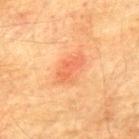The lesion was photographed on a routine skin check and not biopsied; there is no pathology result.
The tile uses cross-polarized illumination.
A region of skin cropped from a whole-body photographic capture, roughly 15 mm wide.
Longest diameter approximately 4 mm.
The lesion is located on the mid back.
A male subject roughly 75 years of age.